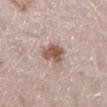Notes:
- biopsy status · total-body-photography surveillance lesion; no biopsy
- body site · the right lower leg
- imaging modality · 15 mm crop, total-body photography
- size · ≈4 mm
- lighting · white-light
- automated metrics · a lesion–skin lightness drop of about 14 and a normalized lesion–skin contrast near 9.5; a lesion-detection confidence of about 100/100
- subject · female, aged 48 to 52